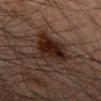Imaged during a routine full-body skin examination; the lesion was not biopsied and no histopathology is available. The lesion's longest dimension is about 6 mm. A 15 mm close-up tile from a total-body photography series done for melanoma screening. Captured under cross-polarized illumination. Located on the right forearm. A male subject roughly 45 years of age.